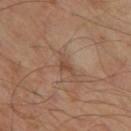Acquisition and patient details:
A male subject aged approximately 45. A lesion tile, about 15 mm wide, cut from a 3D total-body photograph. This is a cross-polarized tile. Automated tile analysis of the lesion measured a footprint of about 3 mm² and an eccentricity of roughly 0.8. The analysis additionally found a lesion color around L≈46 a*≈18 b*≈28 in CIELAB and about 7 CIELAB-L* units darker than the surrounding skin. It also reported a border-irregularity index near 5.5/10 and internal color variation of about 0.5 on a 0–10 scale. The software also gave an automated nevus-likeness rating near 0 out of 100 and lesion-presence confidence of about 95/100. The lesion's longest dimension is about 3 mm. Located on the left thigh.